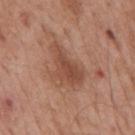No biopsy was performed on this lesion — it was imaged during a full skin examination and was not determined to be concerning. This image is a 15 mm lesion crop taken from a total-body photograph. The lesion is located on the mid back. A male patient aged approximately 55. The lesion's longest dimension is about 5.5 mm. Imaged with white-light lighting.